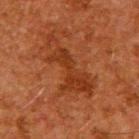About 8.5 mm across. A 15 mm crop from a total-body photograph taken for skin-cancer surveillance. The patient is a male aged 58 to 62. Located on the upper back.